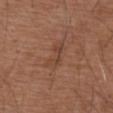Captured during whole-body skin photography for melanoma surveillance; the lesion was not biopsied. From the upper back. Imaged with white-light lighting. The subject is a male aged 73–77. A 15 mm crop from a total-body photograph taken for skin-cancer surveillance.